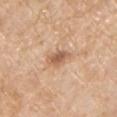Part of a total-body skin-imaging series; this lesion was reviewed on a skin check and was not flagged for biopsy. The subject is a male aged 58 to 62. On the chest. Cropped from a whole-body photographic skin survey; the tile spans about 15 mm.